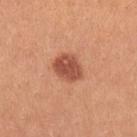Case summary:
* biopsy status: no biopsy performed (imaged during a skin exam)
* patient: female, approximately 25 years of age
* lighting: white-light
* image: ~15 mm crop, total-body skin-cancer survey
* size: ~3.5 mm (longest diameter)
* location: the left upper arm